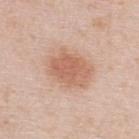No biopsy was performed on this lesion — it was imaged during a full skin examination and was not determined to be concerning. A male patient, aged 38–42. A 15 mm close-up tile from a total-body photography series done for melanoma screening. The recorded lesion diameter is about 5.5 mm. The lesion is on the back. Imaged with white-light lighting. An algorithmic analysis of the crop reported a footprint of about 16 mm², a shape eccentricity near 0.75, and a shape-asymmetry score of about 0.2 (0 = symmetric). It also reported an average lesion color of about L≈62 a*≈22 b*≈31 (CIELAB), about 10 CIELAB-L* units darker than the surrounding skin, and a normalized lesion–skin contrast near 7.